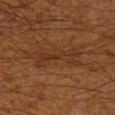Case summary:
* notes — total-body-photography surveillance lesion; no biopsy
* imaging modality — 15 mm crop, total-body photography
* automated metrics — an outline eccentricity of about 0.95 (0 = round, 1 = elongated); an automated nevus-likeness rating near 0 out of 100 and lesion-presence confidence of about 50/100
* diameter — ≈6 mm
* subject — male, approximately 60 years of age
* lighting — cross-polarized illumination
* site — the right lower leg